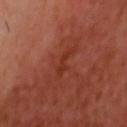A male patient, in their mid- to late 50s. From the head or neck. Automated image analysis of the tile measured an area of roughly 3 mm², an outline eccentricity of about 0.95 (0 = round, 1 = elongated), and a symmetry-axis asymmetry near 0.45. And it measured a lesion–skin lightness drop of about 6. It also reported internal color variation of about 0 on a 0–10 scale and a peripheral color-asymmetry measure near 0. It also reported an automated nevus-likeness rating near 0 out of 100 and lesion-presence confidence of about 95/100. About 3 mm across. A lesion tile, about 15 mm wide, cut from a 3D total-body photograph. Imaged with cross-polarized lighting.